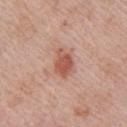Part of a total-body skin-imaging series; this lesion was reviewed on a skin check and was not flagged for biopsy.
Automated image analysis of the tile measured a symmetry-axis asymmetry near 0.25. The software also gave a classifier nevus-likeness of about 90/100.
The tile uses white-light illumination.
A region of skin cropped from a whole-body photographic capture, roughly 15 mm wide.
Measured at roughly 4 mm in maximum diameter.
A male subject in their mid-60s.
On the arm.